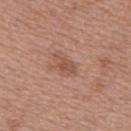Assessment:
This lesion was catalogued during total-body skin photography and was not selected for biopsy.
Image and clinical context:
A female patient, about 40 years old. About 3 mm across. Located on the upper back. A 15 mm close-up extracted from a 3D total-body photography capture.